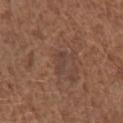Q: What is the lesion's diameter?
A: about 2.5 mm
Q: Patient demographics?
A: female, about 50 years old
Q: How was the tile lit?
A: white-light
Q: Lesion location?
A: the left forearm
Q: What kind of image is this?
A: ~15 mm tile from a whole-body skin photo